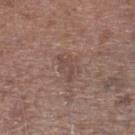Assessment: The lesion was tiled from a total-body skin photograph and was not biopsied. Context: The lesion's longest dimension is about 3.5 mm. Imaged with white-light lighting. Cropped from a whole-body photographic skin survey; the tile spans about 15 mm. The lesion is located on the left lower leg. The subject is a male roughly 75 years of age.